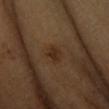Q: Was this lesion biopsied?
A: catalogued during a skin exam; not biopsied
Q: What is the anatomic site?
A: the arm
Q: Illumination type?
A: cross-polarized
Q: Automated lesion metrics?
A: a mean CIELAB color near L≈29 a*≈17 b*≈28, about 7 CIELAB-L* units darker than the surrounding skin, and a normalized lesion–skin contrast near 7; a border-irregularity index near 2/10, internal color variation of about 2 on a 0–10 scale, and peripheral color asymmetry of about 0.5
Q: Patient demographics?
A: female, approximately 55 years of age
Q: What kind of image is this?
A: total-body-photography crop, ~15 mm field of view
Q: How large is the lesion?
A: about 2 mm